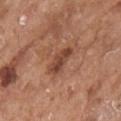Q: Was a biopsy performed?
A: catalogued during a skin exam; not biopsied
Q: What is the imaging modality?
A: ~15 mm crop, total-body skin-cancer survey
Q: What lighting was used for the tile?
A: white-light
Q: Lesion location?
A: the right forearm
Q: Automated lesion metrics?
A: a lesion color around L≈45 a*≈23 b*≈29 in CIELAB, a lesion–skin lightness drop of about 10, and a lesion-to-skin contrast of about 8 (normalized; higher = more distinct); internal color variation of about 3.5 on a 0–10 scale and peripheral color asymmetry of about 1; a classifier nevus-likeness of about 5/100 and lesion-presence confidence of about 100/100
Q: Patient demographics?
A: female, aged 73–77
Q: Lesion size?
A: ~4 mm (longest diameter)